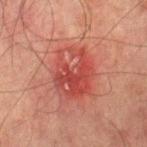Clinical impression:
Imaged during a routine full-body skin examination; the lesion was not biopsied and no histopathology is available.
Clinical summary:
The lesion-visualizer software estimated a lesion area of about 19 mm² and two-axis asymmetry of about 0.15. It also reported an average lesion color of about L≈42 a*≈31 b*≈27 (CIELAB), a lesion–skin lightness drop of about 8, and a lesion-to-skin contrast of about 6.5 (normalized; higher = more distinct). And it measured a border-irregularity index near 2/10, a color-variation rating of about 8.5/10, and peripheral color asymmetry of about 3. The analysis additionally found a nevus-likeness score of about 0/100. The recorded lesion diameter is about 5.5 mm. Imaged with cross-polarized lighting. The subject is a male roughly 75 years of age. A 15 mm close-up tile from a total-body photography series done for melanoma screening. Located on the leg.